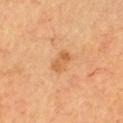Part of a total-body skin-imaging series; this lesion was reviewed on a skin check and was not flagged for biopsy.
The lesion is on the chest.
A lesion tile, about 15 mm wide, cut from a 3D total-body photograph.
A male patient aged 68 to 72.
An algorithmic analysis of the crop reported a footprint of about 4.5 mm² and a shape-asymmetry score of about 0.15 (0 = symmetric). The analysis additionally found a border-irregularity index near 2/10 and radial color variation of about 1. And it measured an automated nevus-likeness rating near 0 out of 100 and lesion-presence confidence of about 100/100.